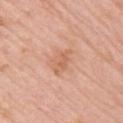The lesion was photographed on a routine skin check and not biopsied; there is no pathology result.
A female patient aged 68 to 72.
The lesion's longest dimension is about 4 mm.
This is a white-light tile.
From the right upper arm.
The total-body-photography lesion software estimated an area of roughly 6.5 mm² and two-axis asymmetry of about 0.4. It also reported a border-irregularity index near 4.5/10, a color-variation rating of about 2.5/10, and a peripheral color-asymmetry measure near 1. And it measured a detector confidence of about 100 out of 100 that the crop contains a lesion.
A region of skin cropped from a whole-body photographic capture, roughly 15 mm wide.